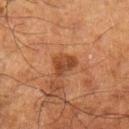A lesion tile, about 15 mm wide, cut from a 3D total-body photograph. The subject is a male about 60 years old. Located on the arm.